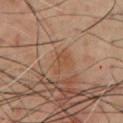Imaged during a routine full-body skin examination; the lesion was not biopsied and no histopathology is available.
Captured under cross-polarized illumination.
The lesion is located on the front of the torso.
A 15 mm close-up extracted from a 3D total-body photography capture.
A male subject aged around 70.